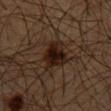The lesion was tiled from a total-body skin photograph and was not biopsied. Captured under cross-polarized illumination. A close-up tile cropped from a whole-body skin photograph, about 15 mm across. The recorded lesion diameter is about 4.5 mm. The subject is a male about 65 years old. Located on the front of the torso.